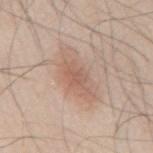biopsy_status: not biopsied; imaged during a skin examination
site: mid back
lighting: white-light
patient:
  sex: male
  age_approx: 45
lesion_size:
  long_diameter_mm_approx: 3.5
image:
  source: total-body photography crop
  field_of_view_mm: 15